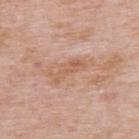Impression:
This lesion was catalogued during total-body skin photography and was not selected for biopsy.
Clinical summary:
On the upper back. A male subject aged 73–77. This is a white-light tile. The lesion-visualizer software estimated border irregularity of about 4.5 on a 0–10 scale, internal color variation of about 2.5 on a 0–10 scale, and a peripheral color-asymmetry measure near 1. The lesion's longest dimension is about 4 mm. A 15 mm close-up tile from a total-body photography series done for melanoma screening.